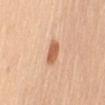A female patient in their mid-50s.
The lesion is located on the right upper arm.
Automated tile analysis of the lesion measured a mean CIELAB color near L≈62 a*≈24 b*≈36, roughly 13 lightness units darker than nearby skin, and a normalized border contrast of about 8.5. The analysis additionally found a border-irregularity rating of about 2/10, a color-variation rating of about 2.5/10, and a peripheral color-asymmetry measure near 1.
A 15 mm close-up tile from a total-body photography series done for melanoma screening.